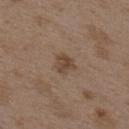Assessment:
This lesion was catalogued during total-body skin photography and was not selected for biopsy.
Acquisition and patient details:
The patient is a female approximately 35 years of age. Measured at roughly 2.5 mm in maximum diameter. A roughly 15 mm field-of-view crop from a total-body skin photograph. Captured under white-light illumination. From the upper back.